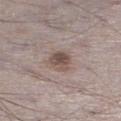Q: Was this lesion biopsied?
A: catalogued during a skin exam; not biopsied
Q: How large is the lesion?
A: about 3 mm
Q: What are the patient's age and sex?
A: male, aged around 70
Q: How was this image acquired?
A: ~15 mm tile from a whole-body skin photo
Q: Illumination type?
A: white-light illumination
Q: Where on the body is the lesion?
A: the leg